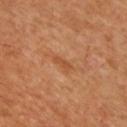Measured at roughly 2.5 mm in maximum diameter.
Cropped from a whole-body photographic skin survey; the tile spans about 15 mm.
The lesion is on the chest.
A male patient, aged 48–52.
Automated tile analysis of the lesion measured internal color variation of about 0 on a 0–10 scale.